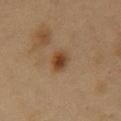Part of a total-body skin-imaging series; this lesion was reviewed on a skin check and was not flagged for biopsy.
Located on the chest.
A male patient approximately 50 years of age.
A 15 mm close-up extracted from a 3D total-body photography capture.
The recorded lesion diameter is about 3 mm.